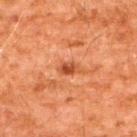The lesion was photographed on a routine skin check and not biopsied; there is no pathology result.
Automated tile analysis of the lesion measured a nevus-likeness score of about 50/100 and lesion-presence confidence of about 100/100.
A 15 mm close-up extracted from a 3D total-body photography capture.
Captured under cross-polarized illumination.
Located on the upper back.
The lesion's longest dimension is about 2.5 mm.
A male patient, roughly 60 years of age.